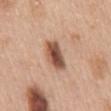size: ≈4 mm
tile lighting: white-light illumination
image source: ~15 mm tile from a whole-body skin photo
location: the mid back
subject: female, approximately 60 years of age